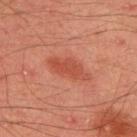workup: total-body-photography surveillance lesion; no biopsy | site: the upper back | lesion diameter: ≈4.5 mm | imaging modality: ~15 mm tile from a whole-body skin photo | subject: male, roughly 45 years of age | automated metrics: a lesion-to-skin contrast of about 6 (normalized; higher = more distinct); a border-irregularity index near 3/10, internal color variation of about 2 on a 0–10 scale, and radial color variation of about 1 | lighting: cross-polarized illumination.